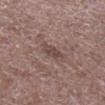biopsy status — catalogued during a skin exam; not biopsied | diameter — ≈3 mm | imaging modality — ~15 mm crop, total-body skin-cancer survey | subject — male, aged 68–72 | TBP lesion metrics — a footprint of about 4 mm², an eccentricity of roughly 0.8, and a symmetry-axis asymmetry near 0.25; a lesion color around L≈44 a*≈17 b*≈19 in CIELAB, a lesion–skin lightness drop of about 9, and a lesion-to-skin contrast of about 7 (normalized; higher = more distinct); a border-irregularity index near 2.5/10, a within-lesion color-variation index near 2.5/10, and a peripheral color-asymmetry measure near 1 | anatomic site — the right lower leg.